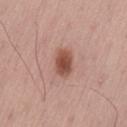Captured during whole-body skin photography for melanoma surveillance; the lesion was not biopsied. The tile uses white-light illumination. A male subject about 55 years old. The lesion is on the lower back. Approximately 3.5 mm at its widest. A 15 mm close-up tile from a total-body photography series done for melanoma screening. The total-body-photography lesion software estimated a footprint of about 6.5 mm², a shape eccentricity near 0.75, and a shape-asymmetry score of about 0.2 (0 = symmetric). It also reported an average lesion color of about L≈50 a*≈24 b*≈27 (CIELAB), about 14 CIELAB-L* units darker than the surrounding skin, and a normalized lesion–skin contrast near 9.5. The analysis additionally found a classifier nevus-likeness of about 100/100 and a lesion-detection confidence of about 100/100.